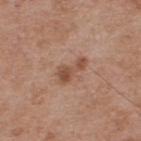Impression:
No biopsy was performed on this lesion — it was imaged during a full skin examination and was not determined to be concerning.
Acquisition and patient details:
Automated image analysis of the tile measured roughly 10 lightness units darker than nearby skin and a lesion-to-skin contrast of about 7.5 (normalized; higher = more distinct). It also reported a classifier nevus-likeness of about 25/100. This is a white-light tile. On the back. The subject is a male aged around 55. Cropped from a whole-body photographic skin survey; the tile spans about 15 mm. Approximately 3.5 mm at its widest.